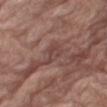Background: This is a white-light tile. Measured at roughly 3 mm in maximum diameter. Located on the right thigh. A male patient about 80 years old. A 15 mm close-up extracted from a 3D total-body photography capture.